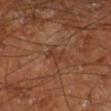| field | value |
|---|---|
| biopsy status | total-body-photography surveillance lesion; no biopsy |
| image source | 15 mm crop, total-body photography |
| illumination | cross-polarized |
| automated metrics | a footprint of about 4.5 mm², a shape eccentricity near 0.75, and two-axis asymmetry of about 0.35; border irregularity of about 4.5 on a 0–10 scale, a within-lesion color-variation index near 2.5/10, and radial color variation of about 1 |
| site | the right lower leg |
| patient | male, about 65 years old |
| lesion size | ~3 mm (longest diameter) |